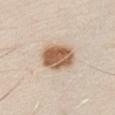This lesion was catalogued during total-body skin photography and was not selected for biopsy.
A 15 mm close-up tile from a total-body photography series done for melanoma screening.
The patient is a male in their 30s.
The lesion is located on the right upper arm.
Imaged with white-light lighting.